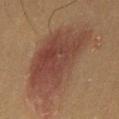Case summary:
• notes · no biopsy performed (imaged during a skin exam)
• subject · female, aged around 50
• image source · 15 mm crop, total-body photography
• body site · the left thigh